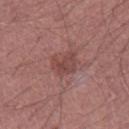Recorded during total-body skin imaging; not selected for excision or biopsy. The patient is a male aged 43 to 47. The lesion is on the left thigh. This image is a 15 mm lesion crop taken from a total-body photograph. The lesion-visualizer software estimated a border-irregularity rating of about 4/10 and internal color variation of about 3 on a 0–10 scale. The analysis additionally found an automated nevus-likeness rating near 0 out of 100 and a detector confidence of about 100 out of 100 that the crop contains a lesion. The recorded lesion diameter is about 3 mm.